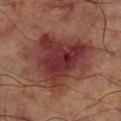Imaged during a routine full-body skin examination; the lesion was not biopsied and no histopathology is available. A male patient approximately 70 years of age. An algorithmic analysis of the crop reported an area of roughly 43 mm², an outline eccentricity of about 0.55 (0 = round, 1 = elongated), and a shape-asymmetry score of about 0.35 (0 = symmetric). The analysis additionally found a mean CIELAB color near L≈28 a*≈21 b*≈19, a lesion–skin lightness drop of about 9, and a normalized lesion–skin contrast near 10. And it measured an automated nevus-likeness rating near 5 out of 100 and a lesion-detection confidence of about 100/100. The lesion is located on the right lower leg. Cropped from a whole-body photographic skin survey; the tile spans about 15 mm. Imaged with cross-polarized lighting. The recorded lesion diameter is about 8.5 mm.